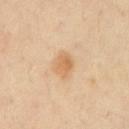  image:
    source: total-body photography crop
    field_of_view_mm: 15
  lesion_size:
    long_diameter_mm_approx: 2.5
  lighting: cross-polarized
  patient:
    sex: male
    age_approx: 50
  site: chest